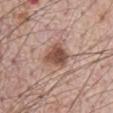Assessment: Captured during whole-body skin photography for melanoma surveillance; the lesion was not biopsied. Clinical summary: A 15 mm crop from a total-body photograph taken for skin-cancer surveillance. The subject is a male aged 68 to 72. The lesion is on the abdomen.